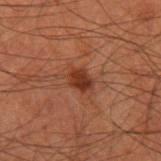workup = no biopsy performed (imaged during a skin exam) | subject = male, approximately 60 years of age | location = the left upper arm | image = ~15 mm tile from a whole-body skin photo | diameter = ≈2.5 mm.